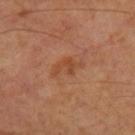Part of a total-body skin-imaging series; this lesion was reviewed on a skin check and was not flagged for biopsy. Approximately 4 mm at its widest. Automated tile analysis of the lesion measured a footprint of about 6 mm², a shape eccentricity near 0.85, and a shape-asymmetry score of about 0.35 (0 = symmetric). It also reported an average lesion color of about L≈45 a*≈24 b*≈34 (CIELAB). The tile uses cross-polarized illumination. A male patient, approximately 65 years of age. This image is a 15 mm lesion crop taken from a total-body photograph.